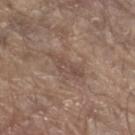Q: Was this lesion biopsied?
A: catalogued during a skin exam; not biopsied
Q: What lighting was used for the tile?
A: white-light
Q: Where on the body is the lesion?
A: the arm
Q: What is the imaging modality?
A: 15 mm crop, total-body photography
Q: Who is the patient?
A: male, roughly 80 years of age
Q: What is the lesion's diameter?
A: ~4 mm (longest diameter)